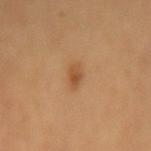The lesion was photographed on a routine skin check and not biopsied; there is no pathology result.
This is a cross-polarized tile.
The patient is female.
This image is a 15 mm lesion crop taken from a total-body photograph.
Measured at roughly 2.5 mm in maximum diameter.
On the mid back.